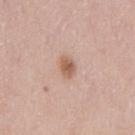- follow-up · total-body-photography surveillance lesion; no biopsy
- subject · male, aged 38–42
- location · the mid back
- tile lighting · white-light
- lesion diameter · ~3 mm (longest diameter)
- image source · ~15 mm tile from a whole-body skin photo